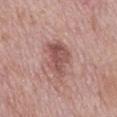This lesion was catalogued during total-body skin photography and was not selected for biopsy. Located on the mid back. A close-up tile cropped from a whole-body skin photograph, about 15 mm across. A male subject, aged approximately 75.